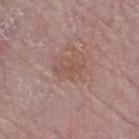follow-up: no biopsy performed (imaged during a skin exam)
size: about 3 mm
illumination: white-light illumination
location: the right thigh
image: 15 mm crop, total-body photography
patient: female, aged 63–67